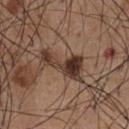Background: The lesion is located on the chest. Automated tile analysis of the lesion measured a lesion area of about 9 mm² and an outline eccentricity of about 0.9 (0 = round, 1 = elongated). And it measured a mean CIELAB color near L≈35 a*≈17 b*≈24, a lesion–skin lightness drop of about 14, and a normalized lesion–skin contrast near 12. The software also gave a border-irregularity index near 8.5/10, a within-lesion color-variation index near 4.5/10, and a peripheral color-asymmetry measure near 1. A roughly 15 mm field-of-view crop from a total-body skin photograph. This is a white-light tile. The lesion's longest dimension is about 5 mm. The patient is a male in their mid- to late 50s.